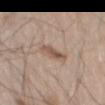Acquisition and patient details:
The lesion is on the left thigh. The tile uses white-light illumination. A region of skin cropped from a whole-body photographic capture, roughly 15 mm wide. The patient is a male roughly 60 years of age. The lesion-visualizer software estimated a footprint of about 7.5 mm² and a shape eccentricity near 0.9. The software also gave a mean CIELAB color near L≈56 a*≈16 b*≈27 and a normalized lesion–skin contrast near 6. It also reported a color-variation rating of about 5/10 and peripheral color asymmetry of about 2. The analysis additionally found an automated nevus-likeness rating near 60 out of 100 and lesion-presence confidence of about 100/100.